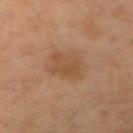Imaged during a routine full-body skin examination; the lesion was not biopsied and no histopathology is available. A roughly 15 mm field-of-view crop from a total-body skin photograph. The subject is a male approximately 60 years of age. Imaged with cross-polarized lighting. About 3.5 mm across. From the right upper arm.